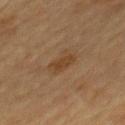notes: catalogued during a skin exam; not biopsied | location: the back | TBP lesion metrics: an average lesion color of about L≈37 a*≈15 b*≈29 (CIELAB), about 6 CIELAB-L* units darker than the surrounding skin, and a lesion-to-skin contrast of about 6 (normalized; higher = more distinct); a classifier nevus-likeness of about 10/100 and a lesion-detection confidence of about 100/100 | illumination: cross-polarized | image: total-body-photography crop, ~15 mm field of view | size: ~3 mm (longest diameter) | subject: female, aged 58 to 62.